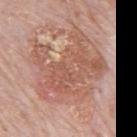Case summary:
- notes — catalogued during a skin exam; not biopsied
- acquisition — ~15 mm crop, total-body skin-cancer survey
- lighting — white-light
- patient — male, approximately 80 years of age
- location — the mid back
- lesion diameter — ≈8.5 mm